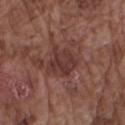Part of a total-body skin-imaging series; this lesion was reviewed on a skin check and was not flagged for biopsy.
Approximately 3.5 mm at its widest.
The total-body-photography lesion software estimated a nevus-likeness score of about 10/100 and a detector confidence of about 100 out of 100 that the crop contains a lesion.
A male subject approximately 75 years of age.
Located on the right upper arm.
A 15 mm crop from a total-body photograph taken for skin-cancer surveillance.